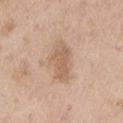Findings:
- image source · ~15 mm tile from a whole-body skin photo
- tile lighting · white-light
- anatomic site · the left upper arm
- subject · male, aged approximately 50
- automated metrics · a lesion color around L≈61 a*≈18 b*≈31 in CIELAB, about 9 CIELAB-L* units darker than the surrounding skin, and a normalized border contrast of about 6.5; an automated nevus-likeness rating near 5 out of 100 and a lesion-detection confidence of about 100/100
- size · ~5 mm (longest diameter)